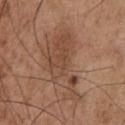Q: Where on the body is the lesion?
A: the chest
Q: Who is the patient?
A: male, aged 53 to 57
Q: What kind of image is this?
A: total-body-photography crop, ~15 mm field of view